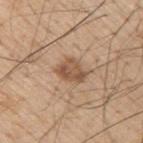This lesion was catalogued during total-body skin photography and was not selected for biopsy. A male patient, in their mid- to late 50s. This image is a 15 mm lesion crop taken from a total-body photograph. Located on the right upper arm. An algorithmic analysis of the crop reported a footprint of about 6.5 mm², an eccentricity of roughly 0.65, and a symmetry-axis asymmetry near 0.3. It also reported a mean CIELAB color near L≈53 a*≈18 b*≈31 and roughly 11 lightness units darker than nearby skin. The tile uses white-light illumination.